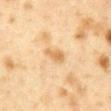Q: Was a biopsy performed?
A: no biopsy performed (imaged during a skin exam)
Q: What is the imaging modality?
A: 15 mm crop, total-body photography
Q: Lesion size?
A: ~3 mm (longest diameter)
Q: Patient demographics?
A: female, aged around 40
Q: Lesion location?
A: the chest
Q: What lighting was used for the tile?
A: cross-polarized illumination